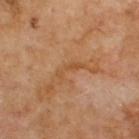  biopsy_status: not biopsied; imaged during a skin examination
  lesion_size:
    long_diameter_mm_approx: 3.5
  lighting: cross-polarized
  site: upper back
  patient:
    sex: male
    age_approx: 70
  image:
    source: total-body photography crop
    field_of_view_mm: 15
  automated_metrics:
    area_mm2_approx: 3.0
    eccentricity: 0.95
    shape_asymmetry: 0.6
    vs_skin_darker_L: 7.0
    vs_skin_contrast_norm: 5.5
    nevus_likeness_0_100: 0